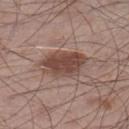Q: Was a biopsy performed?
A: total-body-photography surveillance lesion; no biopsy
Q: Patient demographics?
A: male, in their mid- to late 50s
Q: What lighting was used for the tile?
A: white-light illumination
Q: Lesion size?
A: ~5.5 mm (longest diameter)
Q: What is the imaging modality?
A: total-body-photography crop, ~15 mm field of view
Q: Where on the body is the lesion?
A: the left lower leg
Q: Automated lesion metrics?
A: an area of roughly 13 mm² and a symmetry-axis asymmetry near 0.2; border irregularity of about 2.5 on a 0–10 scale and radial color variation of about 1